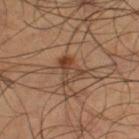{"biopsy_status": "not biopsied; imaged during a skin examination", "patient": {"sex": "male", "age_approx": 60}, "image": {"source": "total-body photography crop", "field_of_view_mm": 15}, "lighting": "cross-polarized", "automated_metrics": {"area_mm2_approx": 5.5, "eccentricity": 0.75, "cielab_L": 41, "cielab_a": 19, "cielab_b": 29, "vs_skin_darker_L": 9.0, "vs_skin_contrast_norm": 7.0, "nevus_likeness_0_100": 20, "lesion_detection_confidence_0_100": 100}, "site": "left thigh", "lesion_size": {"long_diameter_mm_approx": 3.5}}